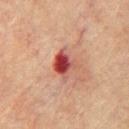Q: Was this lesion biopsied?
A: no biopsy performed (imaged during a skin exam)
Q: What kind of image is this?
A: total-body-photography crop, ~15 mm field of view
Q: What lighting was used for the tile?
A: cross-polarized illumination
Q: How large is the lesion?
A: ~3 mm (longest diameter)
Q: Where on the body is the lesion?
A: the front of the torso
Q: Who is the patient?
A: male, in their mid- to late 70s